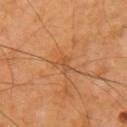Assessment:
Recorded during total-body skin imaging; not selected for excision or biopsy.
Image and clinical context:
Approximately 2.5 mm at its widest. This is a cross-polarized tile. A lesion tile, about 15 mm wide, cut from a 3D total-body photograph. Automated image analysis of the tile measured a border-irregularity index near 6/10, internal color variation of about 1 on a 0–10 scale, and peripheral color asymmetry of about 0.5. Located on the arm. The patient is a male aged approximately 70.